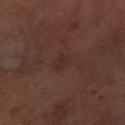- workup · total-body-photography surveillance lesion; no biopsy